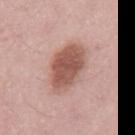{"biopsy_status": "not biopsied; imaged during a skin examination", "patient": {"sex": "male", "age_approx": 55}, "lighting": "white-light", "image": {"source": "total-body photography crop", "field_of_view_mm": 15}, "automated_metrics": {"nevus_likeness_0_100": 100, "lesion_detection_confidence_0_100": 100}}